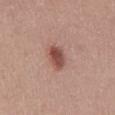The subject is a male about 45 years old.
Automated image analysis of the tile measured an area of roughly 5.5 mm², an eccentricity of roughly 0.7, and two-axis asymmetry of about 0.25. And it measured border irregularity of about 2 on a 0–10 scale and a peripheral color-asymmetry measure near 1.
Cropped from a total-body skin-imaging series; the visible field is about 15 mm.
The lesion is on the front of the torso.
Imaged with white-light lighting.
The lesion's longest dimension is about 3 mm.